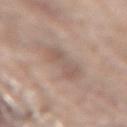Clinical impression:
Recorded during total-body skin imaging; not selected for excision or biopsy.
Image and clinical context:
Located on the lower back. A male patient, aged 78–82. Approximately 5 mm at its widest. A lesion tile, about 15 mm wide, cut from a 3D total-body photograph. This is a white-light tile. An algorithmic analysis of the crop reported a border-irregularity rating of about 5/10 and a within-lesion color-variation index near 3/10. It also reported a detector confidence of about 65 out of 100 that the crop contains a lesion.